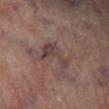Clinical impression:
Imaged during a routine full-body skin examination; the lesion was not biopsied and no histopathology is available.
Context:
The lesion is on the leg. Automated tile analysis of the lesion measured a border-irregularity rating of about 8.5/10, internal color variation of about 3 on a 0–10 scale, and radial color variation of about 1. Cropped from a total-body skin-imaging series; the visible field is about 15 mm. A male patient aged around 75. Longest diameter approximately 5 mm.